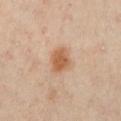Part of a total-body skin-imaging series; this lesion was reviewed on a skin check and was not flagged for biopsy.
The lesion is on the chest.
The total-body-photography lesion software estimated a border-irregularity rating of about 2/10, a color-variation rating of about 3/10, and peripheral color asymmetry of about 1. It also reported a classifier nevus-likeness of about 95/100 and a detector confidence of about 100 out of 100 that the crop contains a lesion.
A male patient, aged around 65.
The lesion's longest dimension is about 3 mm.
A region of skin cropped from a whole-body photographic capture, roughly 15 mm wide.